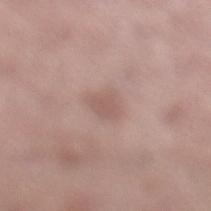Clinical impression: The lesion was photographed on a routine skin check and not biopsied; there is no pathology result. Context: An algorithmic analysis of the crop reported a lesion area of about 5 mm², an eccentricity of roughly 0.6, and two-axis asymmetry of about 0.25. The analysis additionally found a mean CIELAB color near L≈57 a*≈18 b*≈23 and a lesion–skin lightness drop of about 7. Located on the left lower leg. A female patient aged around 50. The lesion's longest dimension is about 3 mm. A close-up tile cropped from a whole-body skin photograph, about 15 mm across. The tile uses white-light illumination.